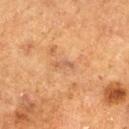* follow-up · total-body-photography surveillance lesion; no biopsy
* illumination · cross-polarized illumination
* patient · male, aged around 75
* imaging modality · total-body-photography crop, ~15 mm field of view
* anatomic site · the right thigh